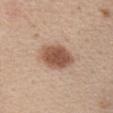follow-up: catalogued during a skin exam; not biopsied
subject: female, aged 33–37
imaging modality: ~15 mm tile from a whole-body skin photo
anatomic site: the chest